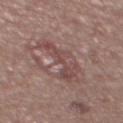Recorded during total-body skin imaging; not selected for excision or biopsy. The total-body-photography lesion software estimated a classifier nevus-likeness of about 0/100 and a detector confidence of about 60 out of 100 that the crop contains a lesion. A 15 mm close-up tile from a total-body photography series done for melanoma screening. Longest diameter approximately 6 mm. The lesion is located on the front of the torso. The subject is a male aged approximately 55.